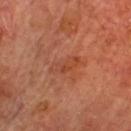image = total-body-photography crop, ~15 mm field of view | patient = male, about 70 years old | diameter = ≈4 mm | anatomic site = the front of the torso.